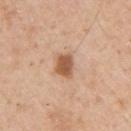Case summary:
- workup · no biopsy performed (imaged during a skin exam)
- subject · male, in their mid-50s
- site · the left upper arm
- image source · ~15 mm crop, total-body skin-cancer survey